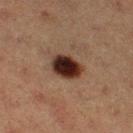Findings:
* subject — female, aged 38 to 42
* site — the right thigh
* image source — 15 mm crop, total-body photography